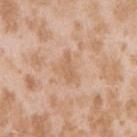Q: Was this lesion biopsied?
A: no biopsy performed (imaged during a skin exam)
Q: Patient demographics?
A: female, aged 23 to 27
Q: What did automated image analysis measure?
A: an automated nevus-likeness rating near 0 out of 100 and lesion-presence confidence of about 100/100
Q: Illumination type?
A: white-light illumination
Q: What kind of image is this?
A: ~15 mm crop, total-body skin-cancer survey
Q: Lesion location?
A: the right upper arm